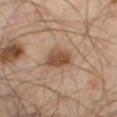{
  "biopsy_status": "not biopsied; imaged during a skin examination",
  "patient": {
    "sex": "male",
    "age_approx": 55
  },
  "site": "left thigh",
  "image": {
    "source": "total-body photography crop",
    "field_of_view_mm": 15
  }
}